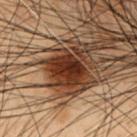Captured during whole-body skin photography for melanoma surveillance; the lesion was not biopsied.
Cropped from a whole-body photographic skin survey; the tile spans about 15 mm.
This is a cross-polarized tile.
Located on the chest.
The patient is a male in their mid-50s.